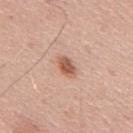Recorded during total-body skin imaging; not selected for excision or biopsy. The lesion is on the mid back. Cropped from a whole-body photographic skin survey; the tile spans about 15 mm. Approximately 3 mm at its widest. The patient is a male aged 53 to 57. Captured under white-light illumination.